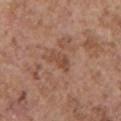Automated image analysis of the tile measured a shape eccentricity near 0.85 and a symmetry-axis asymmetry near 0.45. The analysis additionally found roughly 7 lightness units darker than nearby skin. The software also gave a border-irregularity rating of about 4.5/10 and a within-lesion color-variation index near 2.5/10.
A 15 mm crop from a total-body photograph taken for skin-cancer surveillance.
Measured at roughly 3 mm in maximum diameter.
A female subject in their mid- to late 60s.
Located on the chest.
The tile uses white-light illumination.